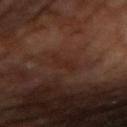Case summary:
• notes — imaged on a skin check; not biopsied
• location — the arm
• patient — male, roughly 55 years of age
• diameter — ~3.5 mm (longest diameter)
• image — ~15 mm crop, total-body skin-cancer survey
• lighting — cross-polarized illumination